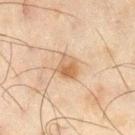| field | value |
|---|---|
| follow-up | total-body-photography surveillance lesion; no biopsy |
| lesion diameter | ~3 mm (longest diameter) |
| image source | ~15 mm crop, total-body skin-cancer survey |
| location | the right thigh |
| lighting | cross-polarized illumination |
| image-analysis metrics | a footprint of about 6 mm², an outline eccentricity of about 0.45 (0 = round, 1 = elongated), and a shape-asymmetry score of about 0.25 (0 = symmetric); a classifier nevus-likeness of about 65/100 and lesion-presence confidence of about 100/100 |
| subject | male, in their mid- to late 40s |